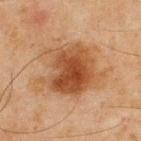The lesion was photographed on a routine skin check and not biopsied; there is no pathology result. A 15 mm close-up extracted from a 3D total-body photography capture. The tile uses cross-polarized illumination. A male patient, in their mid- to late 40s. The lesion is on the upper back. The lesion's longest dimension is about 8 mm.